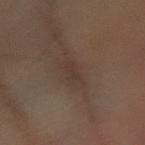{"biopsy_status": "not biopsied; imaged during a skin examination", "patient": {"sex": "female", "age_approx": 55}, "image": {"source": "total-body photography crop", "field_of_view_mm": 15}, "site": "left arm"}